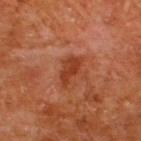Assessment: Captured during whole-body skin photography for melanoma surveillance; the lesion was not biopsied. Context: Captured under cross-polarized illumination. This image is a 15 mm lesion crop taken from a total-body photograph. The lesion is on the upper back. The lesion's longest dimension is about 3.5 mm. The patient is a male approximately 65 years of age.